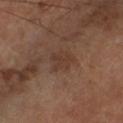This lesion was catalogued during total-body skin photography and was not selected for biopsy. A male patient aged approximately 65. Automated image analysis of the tile measured a border-irregularity rating of about 4/10, a color-variation rating of about 1.5/10, and a peripheral color-asymmetry measure near 0.5. A close-up tile cropped from a whole-body skin photograph, about 15 mm across. The lesion is on the left lower leg. About 3 mm across.